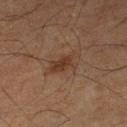This lesion was catalogued during total-body skin photography and was not selected for biopsy. Captured under cross-polarized illumination. A lesion tile, about 15 mm wide, cut from a 3D total-body photograph. Measured at roughly 3.5 mm in maximum diameter. A male patient, roughly 65 years of age. The lesion is on the right lower leg. Automated image analysis of the tile measured an area of roughly 6 mm² and an eccentricity of roughly 0.8. And it measured a border-irregularity index near 4.5/10, internal color variation of about 2.5 on a 0–10 scale, and a peripheral color-asymmetry measure near 1.